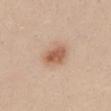Recorded during total-body skin imaging; not selected for excision or biopsy.
Approximately 3.5 mm at its widest.
Captured under white-light illumination.
The total-body-photography lesion software estimated a footprint of about 8.5 mm², a shape eccentricity near 0.65, and two-axis asymmetry of about 0.2. It also reported an average lesion color of about L≈60 a*≈21 b*≈32 (CIELAB), a lesion–skin lightness drop of about 11, and a normalized border contrast of about 8.
A female patient aged 23–27.
The lesion is located on the mid back.
A roughly 15 mm field-of-view crop from a total-body skin photograph.